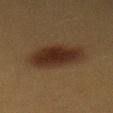Q: Was this lesion biopsied?
A: catalogued during a skin exam; not biopsied
Q: What is the imaging modality?
A: 15 mm crop, total-body photography
Q: Lesion location?
A: the mid back
Q: What is the lesion's diameter?
A: about 6 mm
Q: Patient demographics?
A: female, aged 38 to 42